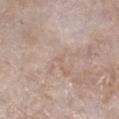Image and clinical context:
On the leg. The tile uses white-light illumination. A female subject, aged 73 to 77. A 15 mm close-up extracted from a 3D total-body photography capture. Measured at roughly 1 mm in maximum diameter.